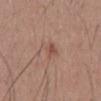Q: Is there a histopathology result?
A: catalogued during a skin exam; not biopsied
Q: Where on the body is the lesion?
A: the mid back
Q: What is the imaging modality?
A: 15 mm crop, total-body photography
Q: Patient demographics?
A: male, aged 28–32